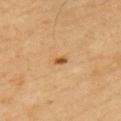No biopsy was performed on this lesion — it was imaged during a full skin examination and was not determined to be concerning.
The subject is a male in their mid- to late 50s.
On the left upper arm.
Imaged with cross-polarized lighting.
About 1.5 mm across.
A lesion tile, about 15 mm wide, cut from a 3D total-body photograph.